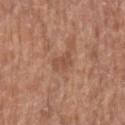Impression:
Captured during whole-body skin photography for melanoma surveillance; the lesion was not biopsied.
Background:
The patient is a female aged around 80. The lesion-visualizer software estimated a lesion area of about 3.5 mm² and a shape eccentricity near 0.85. It also reported border irregularity of about 3.5 on a 0–10 scale, internal color variation of about 1 on a 0–10 scale, and a peripheral color-asymmetry measure near 0. The software also gave an automated nevus-likeness rating near 0 out of 100 and a lesion-detection confidence of about 100/100. The lesion is on the left upper arm. Imaged with white-light lighting. The recorded lesion diameter is about 3 mm. A region of skin cropped from a whole-body photographic capture, roughly 15 mm wide.